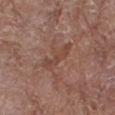From the right thigh. A close-up tile cropped from a whole-body skin photograph, about 15 mm across. Approximately 4.5 mm at its widest. Imaged with white-light lighting. A female patient, aged around 80.